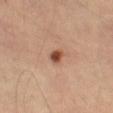The lesion is on the left thigh. The subject is a male approximately 70 years of age. A lesion tile, about 15 mm wide, cut from a 3D total-body photograph. An algorithmic analysis of the crop reported an area of roughly 3 mm², an outline eccentricity of about 0.5 (0 = round, 1 = elongated), and two-axis asymmetry of about 0.2. It also reported a classifier nevus-likeness of about 95/100 and lesion-presence confidence of about 100/100.